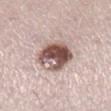{
  "biopsy_status": "not biopsied; imaged during a skin examination",
  "lesion_size": {
    "long_diameter_mm_approx": 4.5
  },
  "image": {
    "source": "total-body photography crop",
    "field_of_view_mm": 15
  },
  "patient": {
    "sex": "female",
    "age_approx": 40
  },
  "site": "right lower leg",
  "lighting": "white-light",
  "automated_metrics": {
    "vs_skin_darker_L": 20.0,
    "border_irregularity_0_10": 2.5,
    "color_variation_0_10": 9.5
  }
}